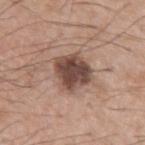Impression: Imaged during a routine full-body skin examination; the lesion was not biopsied and no histopathology is available. Clinical summary: A male patient aged 53 to 57. Cropped from a whole-body photographic skin survey; the tile spans about 15 mm. Approximately 4 mm at its widest.